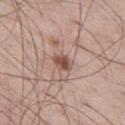<lesion>
<biopsy_status>not biopsied; imaged during a skin examination</biopsy_status>
<lighting>white-light</lighting>
<automated_metrics>
  <area_mm2_approx>4.0</area_mm2_approx>
  <eccentricity>0.7</eccentricity>
  <shape_asymmetry>0.15</shape_asymmetry>
  <lesion_detection_confidence_0_100>100</lesion_detection_confidence_0_100>
</automated_metrics>
<site>left thigh</site>
<image>
  <source>total-body photography crop</source>
  <field_of_view_mm>15</field_of_view_mm>
</image>
<patient>
  <sex>male</sex>
  <age_approx>60</age_approx>
</patient>
</lesion>